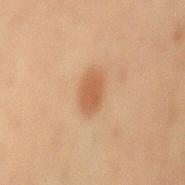- follow-up — no biopsy performed (imaged during a skin exam)
- lesion diameter — about 3.5 mm
- tile lighting — cross-polarized illumination
- subject — male, aged around 70
- image source — ~15 mm tile from a whole-body skin photo
- location — the abdomen